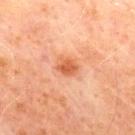Notes:
- workup — no biopsy performed (imaged during a skin exam)
- location — the chest
- patient — male, aged approximately 70
- image — ~15 mm tile from a whole-body skin photo